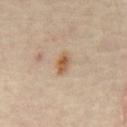Assessment:
The lesion was tiled from a total-body skin photograph and was not biopsied.
Background:
Imaged with cross-polarized lighting. On the chest. A subject approximately 55 years of age. The recorded lesion diameter is about 3 mm. This image is a 15 mm lesion crop taken from a total-body photograph.